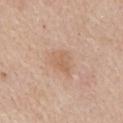This lesion was catalogued during total-body skin photography and was not selected for biopsy.
A 15 mm close-up extracted from a 3D total-body photography capture.
The lesion is on the abdomen.
Imaged with white-light lighting.
A female subject, roughly 65 years of age.